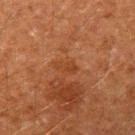- biopsy status: catalogued during a skin exam; not biopsied
- patient: male, in their 60s
- location: the arm
- automated metrics: an area of roughly 3 mm², a shape eccentricity near 0.9, and two-axis asymmetry of about 0.4; a border-irregularity index near 4/10 and a color-variation rating of about 0.5/10
- lighting: cross-polarized illumination
- diameter: about 2.5 mm
- acquisition: ~15 mm tile from a whole-body skin photo